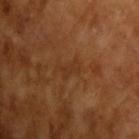{
  "biopsy_status": "not biopsied; imaged during a skin examination",
  "image": {
    "source": "total-body photography crop",
    "field_of_view_mm": 15
  },
  "lighting": "cross-polarized",
  "lesion_size": {
    "long_diameter_mm_approx": 2.5
  },
  "patient": {
    "sex": "male",
    "age_approx": 65
  }
}